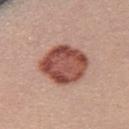Findings:
– workup: catalogued during a skin exam; not biopsied
– image-analysis metrics: an eccentricity of roughly 0.5; an average lesion color of about L≈50 a*≈25 b*≈27 (CIELAB), about 17 CIELAB-L* units darker than the surrounding skin, and a normalized lesion–skin contrast near 11; a classifier nevus-likeness of about 85/100 and a detector confidence of about 100 out of 100 that the crop contains a lesion
– anatomic site: the upper back
– subject: female, in their mid- to late 20s
– lighting: white-light illumination
– image source: 15 mm crop, total-body photography